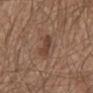workup = no biopsy performed (imaged during a skin exam); image source = ~15 mm crop, total-body skin-cancer survey; body site = the front of the torso; tile lighting = white-light illumination; subject = male, aged approximately 65.